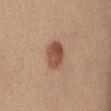location: the chest | imaging modality: ~15 mm tile from a whole-body skin photo | patient: female, approximately 35 years of age.